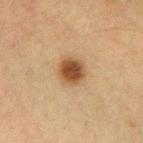The lesion was tiled from a total-body skin photograph and was not biopsied. Captured under cross-polarized illumination. A female subject, in their mid-50s. A 15 mm close-up extracted from a 3D total-body photography capture. The recorded lesion diameter is about 3 mm. Located on the left forearm.